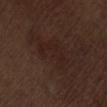notes: imaged on a skin check; not biopsied | image: ~15 mm tile from a whole-body skin photo | patient: male, aged approximately 70 | anatomic site: the lower back | lesion size: about 5 mm | lighting: white-light illumination | TBP lesion metrics: a shape eccentricity near 0.8; about 3 CIELAB-L* units darker than the surrounding skin and a lesion-to-skin contrast of about 4.5 (normalized; higher = more distinct).